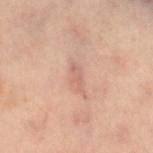Captured during whole-body skin photography for melanoma surveillance; the lesion was not biopsied.
The patient is a female approximately 60 years of age.
A 15 mm crop from a total-body photograph taken for skin-cancer surveillance.
Automated tile analysis of the lesion measured a border-irregularity index near 3.5/10 and a color-variation rating of about 0.5/10. The software also gave a classifier nevus-likeness of about 0/100 and lesion-presence confidence of about 100/100.
The tile uses cross-polarized illumination.
From the leg.
The recorded lesion diameter is about 2.5 mm.